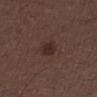workup: catalogued during a skin exam; not biopsied
site: the left thigh
size: ~2.5 mm (longest diameter)
imaging modality: ~15 mm crop, total-body skin-cancer survey
automated lesion analysis: a mean CIELAB color near L≈27 a*≈16 b*≈18, a lesion–skin lightness drop of about 7, and a lesion-to-skin contrast of about 7.5 (normalized; higher = more distinct); a border-irregularity index near 2/10, a color-variation rating of about 2.5/10, and a peripheral color-asymmetry measure near 1
patient: male, aged approximately 50
tile lighting: white-light